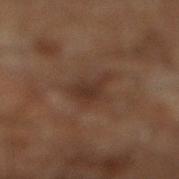This lesion was catalogued during total-body skin photography and was not selected for biopsy. Located on the leg. The lesion's longest dimension is about 3.5 mm. The subject is a male about 60 years old. A 15 mm crop from a total-body photograph taken for skin-cancer surveillance. Imaged with cross-polarized lighting.